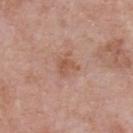This lesion was catalogued during total-body skin photography and was not selected for biopsy. Measured at roughly 2.5 mm in maximum diameter. A male patient aged 53–57. Cropped from a whole-body photographic skin survey; the tile spans about 15 mm. The lesion is on the chest. This is a white-light tile.